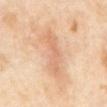No biopsy was performed on this lesion — it was imaged during a full skin examination and was not determined to be concerning. A female patient roughly 50 years of age. Located on the front of the torso. Cropped from a total-body skin-imaging series; the visible field is about 15 mm.